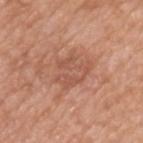notes: total-body-photography surveillance lesion; no biopsy
subject: male, about 50 years old
diameter: ~4.5 mm (longest diameter)
anatomic site: the upper back
imaging modality: ~15 mm crop, total-body skin-cancer survey
tile lighting: white-light
image-analysis metrics: a footprint of about 11 mm², an eccentricity of roughly 0.65, and a symmetry-axis asymmetry near 0.35; border irregularity of about 4.5 on a 0–10 scale, a color-variation rating of about 3/10, and a peripheral color-asymmetry measure near 1; a classifier nevus-likeness of about 0/100 and lesion-presence confidence of about 100/100